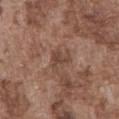The lesion was tiled from a total-body skin photograph and was not biopsied.
A male subject, approximately 75 years of age.
Longest diameter approximately 2.5 mm.
The lesion is on the abdomen.
A close-up tile cropped from a whole-body skin photograph, about 15 mm across.
Imaged with white-light lighting.